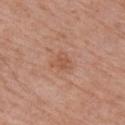Q: Is there a histopathology result?
A: no biopsy performed (imaged during a skin exam)
Q: Where on the body is the lesion?
A: the right upper arm
Q: Patient demographics?
A: male, in their mid- to late 60s
Q: Automated lesion metrics?
A: a lesion area of about 4.5 mm², a shape eccentricity near 0.6, and a shape-asymmetry score of about 0.45 (0 = symmetric); a lesion color around L≈54 a*≈23 b*≈32 in CIELAB, a lesion–skin lightness drop of about 7, and a lesion-to-skin contrast of about 5 (normalized; higher = more distinct); a border-irregularity rating of about 4/10, a color-variation rating of about 1.5/10, and a peripheral color-asymmetry measure near 0.5; a nevus-likeness score of about 0/100 and lesion-presence confidence of about 100/100
Q: Lesion size?
A: ≈3 mm
Q: How was the tile lit?
A: white-light illumination
Q: What kind of image is this?
A: 15 mm crop, total-body photography